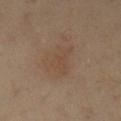Clinical impression:
Part of a total-body skin-imaging series; this lesion was reviewed on a skin check and was not flagged for biopsy.
Clinical summary:
From the leg. The lesion-visualizer software estimated an area of roughly 8.5 mm² and a shape-asymmetry score of about 0.45 (0 = symmetric). The analysis additionally found a normalized border contrast of about 4. A close-up tile cropped from a whole-body skin photograph, about 15 mm across. A female subject, approximately 40 years of age. This is a cross-polarized tile.